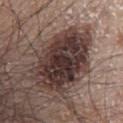notes=total-body-photography surveillance lesion; no biopsy | subject=male, in their mid- to late 60s | lesion diameter=about 8 mm | imaging modality=~15 mm tile from a whole-body skin photo | site=the abdomen.